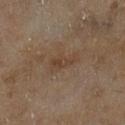The lesion was photographed on a routine skin check and not biopsied; there is no pathology result.
A roughly 15 mm field-of-view crop from a total-body skin photograph.
Captured under cross-polarized illumination.
Located on the leg.
Longest diameter approximately 4 mm.
A female patient aged approximately 60.
The total-body-photography lesion software estimated an area of roughly 6 mm², an eccentricity of roughly 0.9, and a shape-asymmetry score of about 0.35 (0 = symmetric). The analysis additionally found border irregularity of about 4.5 on a 0–10 scale, a within-lesion color-variation index near 2.5/10, and radial color variation of about 1.